A male subject aged 48–52; from the left upper arm; this image is a 15 mm lesion crop taken from a total-body photograph; imaged with white-light lighting.
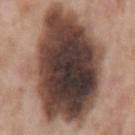The lesion was biopsied, and histopathology showed an atypical melanocytic neoplasm, classified as an indeterminate (borderline) lesion.